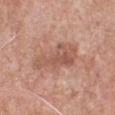Q: Was this lesion biopsied?
A: no biopsy performed (imaged during a skin exam)
Q: Lesion location?
A: the chest
Q: How was the tile lit?
A: white-light
Q: What did automated image analysis measure?
A: a mean CIELAB color near L≈55 a*≈23 b*≈28, about 9 CIELAB-L* units darker than the surrounding skin, and a lesion-to-skin contrast of about 6.5 (normalized; higher = more distinct)
Q: Patient demographics?
A: male, roughly 60 years of age
Q: How was this image acquired?
A: ~15 mm tile from a whole-body skin photo
Q: Lesion size?
A: about 6 mm